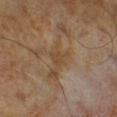No biopsy was performed on this lesion — it was imaged during a full skin examination and was not determined to be concerning. The patient is a male about 65 years old. The tile uses cross-polarized illumination. Cropped from a whole-body photographic skin survey; the tile spans about 15 mm. The lesion-visualizer software estimated a lesion area of about 4 mm² and two-axis asymmetry of about 0.4. The software also gave an average lesion color of about L≈44 a*≈15 b*≈31 (CIELAB) and a normalized border contrast of about 5. And it measured a border-irregularity index near 4.5/10 and peripheral color asymmetry of about 0.5. The lesion's longest dimension is about 2.5 mm.